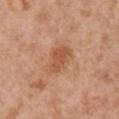This lesion was catalogued during total-body skin photography and was not selected for biopsy.
The subject is a female aged around 55.
The lesion is located on the leg.
Automated image analysis of the tile measured an area of roughly 9 mm², an eccentricity of roughly 0.75, and two-axis asymmetry of about 0.25. It also reported a mean CIELAB color near L≈55 a*≈24 b*≈35, about 8 CIELAB-L* units darker than the surrounding skin, and a lesion-to-skin contrast of about 6.5 (normalized; higher = more distinct). The software also gave a nevus-likeness score of about 15/100 and a detector confidence of about 100 out of 100 that the crop contains a lesion.
About 4 mm across.
Imaged with cross-polarized lighting.
Cropped from a total-body skin-imaging series; the visible field is about 15 mm.